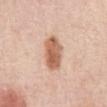Q: Was a biopsy performed?
A: imaged on a skin check; not biopsied
Q: What kind of image is this?
A: ~15 mm tile from a whole-body skin photo
Q: Who is the patient?
A: female, aged 63 to 67
Q: What is the anatomic site?
A: the front of the torso